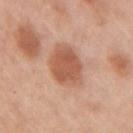Q: Is there a histopathology result?
A: no biopsy performed (imaged during a skin exam)
Q: How was the tile lit?
A: white-light
Q: Where on the body is the lesion?
A: the right upper arm
Q: Patient demographics?
A: female, in their mid- to late 40s
Q: What is the lesion's diameter?
A: ≈4.5 mm
Q: How was this image acquired?
A: total-body-photography crop, ~15 mm field of view